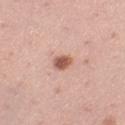Located on the left thigh. A 15 mm crop from a total-body photograph taken for skin-cancer surveillance. The subject is a female roughly 35 years of age. The tile uses white-light illumination. The recorded lesion diameter is about 2.5 mm. Automated tile analysis of the lesion measured an average lesion color of about L≈57 a*≈24 b*≈28 (CIELAB), about 15 CIELAB-L* units darker than the surrounding skin, and a normalized lesion–skin contrast near 9.5. The analysis additionally found a border-irregularity index near 2/10, internal color variation of about 2 on a 0–10 scale, and peripheral color asymmetry of about 0.5.